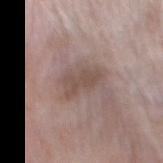Clinical summary: The lesion's longest dimension is about 4.5 mm. The tile uses white-light illumination. On the mid back. A male patient aged 53 to 57. A region of skin cropped from a whole-body photographic capture, roughly 15 mm wide.